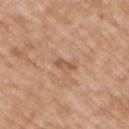Q: Is there a histopathology result?
A: catalogued during a skin exam; not biopsied
Q: Who is the patient?
A: male, aged around 50
Q: What is the anatomic site?
A: the back
Q: How was this image acquired?
A: total-body-photography crop, ~15 mm field of view
Q: How large is the lesion?
A: about 2.5 mm